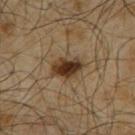notes — catalogued during a skin exam; not biopsied | body site — the upper back | image source — total-body-photography crop, ~15 mm field of view | illumination — cross-polarized illumination | patient — male, aged approximately 65 | size — about 3.5 mm | image-analysis metrics — a shape eccentricity near 0.75 and a symmetry-axis asymmetry near 0.15; border irregularity of about 2 on a 0–10 scale and a peripheral color-asymmetry measure near 2; a classifier nevus-likeness of about 95/100.